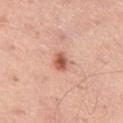follow-up = catalogued during a skin exam; not biopsied
imaging modality = 15 mm crop, total-body photography
lesion diameter = about 2.5 mm
anatomic site = the left thigh
illumination = white-light
subject = male, aged 48 to 52